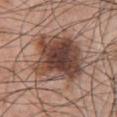follow-up: catalogued during a skin exam; not biopsied
tile lighting: white-light illumination
acquisition: total-body-photography crop, ~15 mm field of view
location: the chest
size: ≈7 mm
patient: male, about 70 years old
TBP lesion metrics: a lesion–skin lightness drop of about 15 and a normalized lesion–skin contrast near 11; border irregularity of about 2.5 on a 0–10 scale, internal color variation of about 8 on a 0–10 scale, and radial color variation of about 2.5